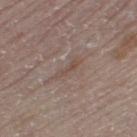The lesion was photographed on a routine skin check and not biopsied; there is no pathology result.